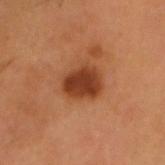{
  "site": "head or neck",
  "patient": {
    "sex": "male",
    "age_approx": 55
  },
  "image": {
    "source": "total-body photography crop",
    "field_of_view_mm": 15
  },
  "automated_metrics": {
    "vs_skin_darker_L": 13.0,
    "nevus_likeness_0_100": 95,
    "lesion_detection_confidence_0_100": 100
  },
  "lesion_size": {
    "long_diameter_mm_approx": 4.0
  },
  "lighting": "cross-polarized"
}